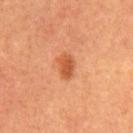Findings:
- biopsy status: catalogued during a skin exam; not biopsied
- imaging modality: ~15 mm tile from a whole-body skin photo
- patient: male, in their mid- to late 60s
- size: about 3 mm
- anatomic site: the abdomen